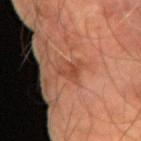The lesion was photographed on a routine skin check and not biopsied; there is no pathology result. The subject is a male about 60 years old. Located on the arm. Automated tile analysis of the lesion measured a footprint of about 4.5 mm² and a symmetry-axis asymmetry near 0.45. And it measured a border-irregularity rating of about 5/10, a within-lesion color-variation index near 3/10, and radial color variation of about 1. The software also gave a classifier nevus-likeness of about 0/100 and a lesion-detection confidence of about 100/100. Cropped from a total-body skin-imaging series; the visible field is about 15 mm. Captured under cross-polarized illumination. The recorded lesion diameter is about 3 mm.